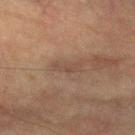Findings:
* workup · total-body-photography surveillance lesion; no biopsy
* subject · male, in their mid-70s
* lesion size · ≈3 mm
* image · ~15 mm crop, total-body skin-cancer survey
* site · the left forearm
* illumination · cross-polarized illumination